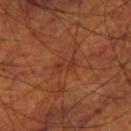Findings:
• notes · no biopsy performed (imaged during a skin exam)
• image · ~15 mm crop, total-body skin-cancer survey
• lighting · cross-polarized illumination
• lesion size · about 3 mm
• subject · male, approximately 70 years of age
• site · the leg
• automated metrics · a lesion–skin lightness drop of about 5 and a normalized lesion–skin contrast near 5; a nevus-likeness score of about 0/100 and a lesion-detection confidence of about 90/100